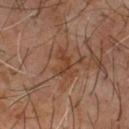Q: Is there a histopathology result?
A: imaged on a skin check; not biopsied
Q: What is the anatomic site?
A: the chest
Q: What kind of image is this?
A: ~15 mm tile from a whole-body skin photo
Q: Who is the patient?
A: male, aged approximately 60